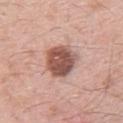Notes:
* workup · imaged on a skin check; not biopsied
* diameter · ≈4.5 mm
* image · total-body-photography crop, ~15 mm field of view
* patient · male, aged approximately 25
* lighting · white-light
* automated lesion analysis · an area of roughly 13 mm²; a mean CIELAB color near L≈53 a*≈22 b*≈25; a peripheral color-asymmetry measure near 1.5
* anatomic site · the upper back